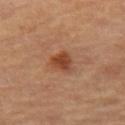follow-up — imaged on a skin check; not biopsied.